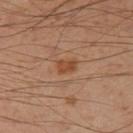notes: imaged on a skin check; not biopsied | acquisition: total-body-photography crop, ~15 mm field of view | diameter: ≈2.5 mm | subject: male, in their mid-50s | location: the right thigh | illumination: cross-polarized | TBP lesion metrics: a footprint of about 4 mm²; a nevus-likeness score of about 65/100.